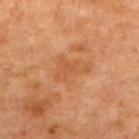{
  "biopsy_status": "not biopsied; imaged during a skin examination",
  "image": {
    "source": "total-body photography crop",
    "field_of_view_mm": 15
  },
  "site": "upper back",
  "patient": {
    "age_approx": 65
  }
}